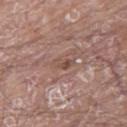{"biopsy_status": "not biopsied; imaged during a skin examination", "patient": {"sex": "male", "age_approx": 80}, "site": "right thigh", "image": {"source": "total-body photography crop", "field_of_view_mm": 15}}